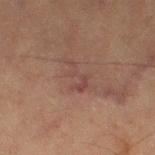Cropped from a total-body skin-imaging series; the visible field is about 15 mm.
A female subject roughly 60 years of age.
Measured at roughly 3.5 mm in maximum diameter.
On the left thigh.
Imaged with cross-polarized lighting.